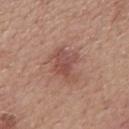Part of a total-body skin-imaging series; this lesion was reviewed on a skin check and was not flagged for biopsy.
A male subject aged around 50.
Longest diameter approximately 4.5 mm.
The lesion-visualizer software estimated a border-irregularity rating of about 4/10 and a peripheral color-asymmetry measure near 1.5.
A region of skin cropped from a whole-body photographic capture, roughly 15 mm wide.
The tile uses white-light illumination.
The lesion is on the mid back.